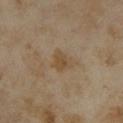| feature | finding |
|---|---|
| follow-up | imaged on a skin check; not biopsied |
| site | the arm |
| illumination | cross-polarized illumination |
| subject | female, aged around 35 |
| image source | ~15 mm crop, total-body skin-cancer survey |